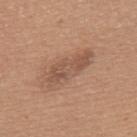Clinical impression:
The lesion was photographed on a routine skin check and not biopsied; there is no pathology result.
Clinical summary:
A 15 mm close-up tile from a total-body photography series done for melanoma screening. On the upper back. A female patient in their mid- to late 50s. The tile uses white-light illumination. About 6 mm across.